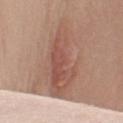Part of a total-body skin-imaging series; this lesion was reviewed on a skin check and was not flagged for biopsy.
A roughly 15 mm field-of-view crop from a total-body skin photograph.
The lesion is located on the right upper arm.
A female subject, aged 48 to 52.